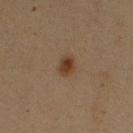workup: total-body-photography surveillance lesion; no biopsy | anatomic site: the right upper arm | TBP lesion metrics: a lesion–skin lightness drop of about 9 and a lesion-to-skin contrast of about 9.5 (normalized; higher = more distinct); border irregularity of about 1.5 on a 0–10 scale and peripheral color asymmetry of about 1; a classifier nevus-likeness of about 100/100 and a lesion-detection confidence of about 100/100 | patient: female, roughly 40 years of age | tile lighting: cross-polarized illumination | image: ~15 mm tile from a whole-body skin photo | lesion size: about 2.5 mm.